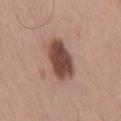Notes:
- notes: total-body-photography surveillance lesion; no biopsy
- image-analysis metrics: a lesion area of about 13 mm² and a shape eccentricity near 0.8; a lesion color around L≈46 a*≈21 b*≈24 in CIELAB and a normalized lesion–skin contrast near 11.5; a border-irregularity index near 2/10, a color-variation rating of about 4/10, and peripheral color asymmetry of about 1; an automated nevus-likeness rating near 85 out of 100 and lesion-presence confidence of about 100/100
- illumination: white-light illumination
- subject: male, in their mid-60s
- anatomic site: the back
- image source: 15 mm crop, total-body photography
- diameter: about 5 mm